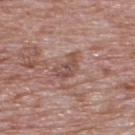Captured during whole-body skin photography for melanoma surveillance; the lesion was not biopsied. The tile uses white-light illumination. The lesion is located on the back. A close-up tile cropped from a whole-body skin photograph, about 15 mm across. A male patient roughly 70 years of age. About 3.5 mm across.